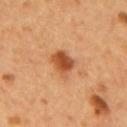Clinical impression:
Captured during whole-body skin photography for melanoma surveillance; the lesion was not biopsied.
Background:
A close-up tile cropped from a whole-body skin photograph, about 15 mm across. A male patient about 55 years old. The lesion is on the mid back. Imaged with cross-polarized lighting. The lesion-visualizer software estimated a border-irregularity rating of about 3/10, a color-variation rating of about 4/10, and radial color variation of about 1. And it measured a nevus-likeness score of about 95/100 and lesion-presence confidence of about 100/100.